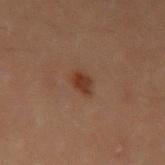Assessment:
This lesion was catalogued during total-body skin photography and was not selected for biopsy.
Acquisition and patient details:
Imaged with cross-polarized lighting. The lesion-visualizer software estimated a mean CIELAB color near L≈27 a*≈17 b*≈23, about 8 CIELAB-L* units darker than the surrounding skin, and a normalized border contrast of about 8.5. The software also gave an automated nevus-likeness rating near 90 out of 100. The recorded lesion diameter is about 2.5 mm. From the left upper arm. Cropped from a total-body skin-imaging series; the visible field is about 15 mm. The subject is a female approximately 20 years of age.